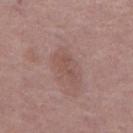The lesion was photographed on a routine skin check and not biopsied; there is no pathology result. A close-up tile cropped from a whole-body skin photograph, about 15 mm across. The subject is a male approximately 70 years of age. The lesion is on the left thigh. This is a white-light tile.